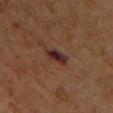| key | value |
|---|---|
| biopsy status | catalogued during a skin exam; not biopsied |
| image source | ~15 mm crop, total-body skin-cancer survey |
| anatomic site | the chest |
| patient | male, about 65 years old |
| image-analysis metrics | a lesion color around L≈23 a*≈17 b*≈17 in CIELAB, a lesion–skin lightness drop of about 8, and a normalized border contrast of about 11.5; a border-irregularity rating of about 3/10, internal color variation of about 5.5 on a 0–10 scale, and peripheral color asymmetry of about 1.5; a classifier nevus-likeness of about 0/100 and a lesion-detection confidence of about 100/100 |
| illumination | cross-polarized illumination |
| diameter | ~3.5 mm (longest diameter) |